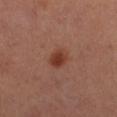Findings:
– notes: no biopsy performed (imaged during a skin exam)
– imaging modality: 15 mm crop, total-body photography
– subject: female
– anatomic site: the right thigh
– lighting: cross-polarized illumination
– automated metrics: an area of roughly 5.5 mm²; an average lesion color of about L≈39 a*≈24 b*≈29 (CIELAB), a lesion–skin lightness drop of about 9, and a normalized border contrast of about 8; border irregularity of about 1.5 on a 0–10 scale, a within-lesion color-variation index near 3/10, and radial color variation of about 1